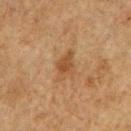A lesion tile, about 15 mm wide, cut from a 3D total-body photograph. The lesion is located on the chest. About 3.5 mm across. A male patient in their mid- to late 70s.